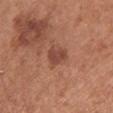Part of a total-body skin-imaging series; this lesion was reviewed on a skin check and was not flagged for biopsy.
The lesion is on the chest.
An algorithmic analysis of the crop reported an automated nevus-likeness rating near 40 out of 100 and lesion-presence confidence of about 100/100.
Cropped from a whole-body photographic skin survey; the tile spans about 15 mm.
The patient is a female aged 63–67.